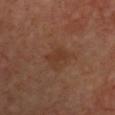Impression: This lesion was catalogued during total-body skin photography and was not selected for biopsy. Clinical summary: The lesion-visualizer software estimated a footprint of about 4.5 mm², an outline eccentricity of about 0.8 (0 = round, 1 = elongated), and a symmetry-axis asymmetry near 0.3. The analysis additionally found border irregularity of about 3 on a 0–10 scale, a within-lesion color-variation index near 1.5/10, and radial color variation of about 0.5. The lesion is located on the chest. About 3 mm across. A female patient, aged approximately 40. Captured under cross-polarized illumination. This image is a 15 mm lesion crop taken from a total-body photograph.